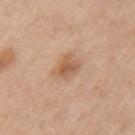No biopsy was performed on this lesion — it was imaged during a full skin examination and was not determined to be concerning.
A 15 mm close-up tile from a total-body photography series done for melanoma screening.
Imaged with white-light lighting.
On the left upper arm.
About 3 mm across.
Automated tile analysis of the lesion measured an average lesion color of about L≈58 a*≈20 b*≈34 (CIELAB) and a normalized lesion–skin contrast near 7. And it measured radial color variation of about 1.
A male subject, approximately 70 years of age.